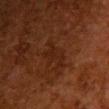| key | value |
|---|---|
| follow-up | catalogued during a skin exam; not biopsied |
| patient | female, aged around 50 |
| image-analysis metrics | a footprint of about 7 mm², a shape eccentricity near 0.85, and a symmetry-axis asymmetry near 0.45; roughly 4 lightness units darker than nearby skin and a normalized border contrast of about 5.5; a border-irregularity rating of about 7/10 and a within-lesion color-variation index near 1.5/10 |
| image | ~15 mm crop, total-body skin-cancer survey |
| size | ≈4.5 mm |
| location | the chest |